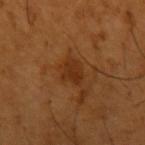Impression:
Captured during whole-body skin photography for melanoma surveillance; the lesion was not biopsied.
Context:
On the left forearm. Captured under cross-polarized illumination. Cropped from a total-body skin-imaging series; the visible field is about 15 mm. The lesion-visualizer software estimated a shape eccentricity near 0.7 and two-axis asymmetry of about 0.25. And it measured a mean CIELAB color near L≈31 a*≈23 b*≈34 and roughly 7 lightness units darker than nearby skin. And it measured a detector confidence of about 100 out of 100 that the crop contains a lesion. A male subject, in their 50s. Measured at roughly 3 mm in maximum diameter.